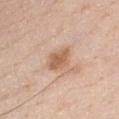Case summary:
- follow-up: catalogued during a skin exam; not biopsied
- subject: male, about 60 years old
- location: the chest
- image source: ~15 mm tile from a whole-body skin photo